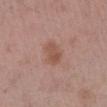No biopsy was performed on this lesion — it was imaged during a full skin examination and was not determined to be concerning.
A male subject, about 55 years old.
This image is a 15 mm lesion crop taken from a total-body photograph.
Located on the left lower leg.